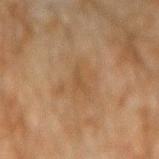Q: Is there a histopathology result?
A: imaged on a skin check; not biopsied
Q: Who is the patient?
A: male, aged 43–47
Q: Illumination type?
A: cross-polarized illumination
Q: What is the imaging modality?
A: total-body-photography crop, ~15 mm field of view
Q: Lesion location?
A: the left forearm